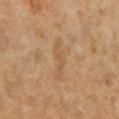Captured during whole-body skin photography for melanoma surveillance; the lesion was not biopsied.
The lesion is on the left lower leg.
The recorded lesion diameter is about 4.5 mm.
Cropped from a total-body skin-imaging series; the visible field is about 15 mm.
A female patient aged 53–57.